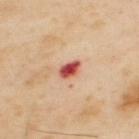{
  "biopsy_status": "not biopsied; imaged during a skin examination",
  "automated_metrics": {
    "area_mm2_approx": 4.0,
    "eccentricity": 0.75,
    "nevus_likeness_0_100": 0,
    "lesion_detection_confidence_0_100": 100
  },
  "patient": {
    "sex": "male",
    "age_approx": 55
  },
  "site": "upper back",
  "lesion_size": {
    "long_diameter_mm_approx": 2.5
  },
  "image": {
    "source": "total-body photography crop",
    "field_of_view_mm": 15
  }
}